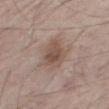Clinical impression: Recorded during total-body skin imaging; not selected for excision or biopsy. Clinical summary: A male subject approximately 65 years of age. Longest diameter approximately 4.5 mm. This is a white-light tile. A roughly 15 mm field-of-view crop from a total-body skin photograph. The lesion is on the right thigh.